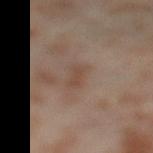Clinical impression: Captured during whole-body skin photography for melanoma surveillance; the lesion was not biopsied. Acquisition and patient details: A region of skin cropped from a whole-body photographic capture, roughly 15 mm wide. Measured at roughly 3 mm in maximum diameter. Captured under cross-polarized illumination. The patient is a female in their mid- to late 50s. Automated image analysis of the tile measured a lesion area of about 2.5 mm², an outline eccentricity of about 0.95 (0 = round, 1 = elongated), and a shape-asymmetry score of about 0.4 (0 = symmetric). The software also gave a mean CIELAB color near L≈46 a*≈16 b*≈27 and a lesion-to-skin contrast of about 6 (normalized; higher = more distinct). The analysis additionally found a border-irregularity rating of about 4.5/10 and a color-variation rating of about 0/10. The software also gave an automated nevus-likeness rating near 0 out of 100 and lesion-presence confidence of about 100/100. On the leg.